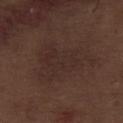The lesion was tiled from a total-body skin photograph and was not biopsied. The lesion is on the abdomen. The subject is a male aged around 70. A 15 mm crop from a total-body photograph taken for skin-cancer surveillance. Imaged with white-light lighting. Automated image analysis of the tile measured a lesion color around L≈27 a*≈15 b*≈19 in CIELAB, about 4 CIELAB-L* units darker than the surrounding skin, and a normalized lesion–skin contrast near 4.5. And it measured a lesion-detection confidence of about 100/100.